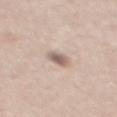biopsy status = no biopsy performed (imaged during a skin exam)
illumination = white-light illumination
image = ~15 mm tile from a whole-body skin photo
lesion diameter = about 2.5 mm
site = the back
subject = male, in their mid-40s